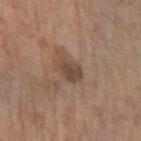Part of a total-body skin-imaging series; this lesion was reviewed on a skin check and was not flagged for biopsy. From the left forearm. The lesion-visualizer software estimated an eccentricity of roughly 0.65 and two-axis asymmetry of about 0.25. And it measured a nevus-likeness score of about 0/100. A close-up tile cropped from a whole-body skin photograph, about 15 mm across. A female patient, aged 58–62.